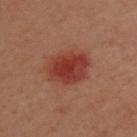No biopsy was performed on this lesion — it was imaged during a full skin examination and was not determined to be concerning. The patient is a male aged around 40. A 15 mm crop from a total-body photograph taken for skin-cancer surveillance. Captured under cross-polarized illumination. Located on the chest. Measured at roughly 5 mm in maximum diameter.